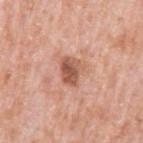<tbp_lesion>
  <biopsy_status>not biopsied; imaged during a skin examination</biopsy_status>
  <patient>
    <sex>male</sex>
    <age_approx>80</age_approx>
  </patient>
  <image>
    <source>total-body photography crop</source>
    <field_of_view_mm>15</field_of_view_mm>
  </image>
  <lesion_size>
    <long_diameter_mm_approx>3.5</long_diameter_mm_approx>
  </lesion_size>
  <lighting>white-light</lighting>
  <site>left upper arm</site>
  <automated_metrics>
    <cielab_L>56</cielab_L>
    <cielab_a>25</cielab_a>
    <cielab_b>30</cielab_b>
    <vs_skin_darker_L>13.0</vs_skin_darker_L>
    <vs_skin_contrast_norm>8.5</vs_skin_contrast_norm>
    <border_irregularity_0_10>2.5</border_irregularity_0_10>
    <color_variation_0_10>5.0</color_variation_0_10>
    <peripheral_color_asymmetry>1.5</peripheral_color_asymmetry>
  </automated_metrics>
</tbp_lesion>